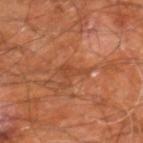Q: Is there a histopathology result?
A: catalogued during a skin exam; not biopsied
Q: Patient demographics?
A: male, aged 58–62
Q: What is the imaging modality?
A: 15 mm crop, total-body photography
Q: Lesion size?
A: ~3.5 mm (longest diameter)
Q: Where on the body is the lesion?
A: the right leg
Q: How was the tile lit?
A: cross-polarized